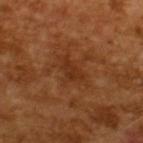Clinical impression:
The lesion was tiled from a total-body skin photograph and was not biopsied.
Acquisition and patient details:
Captured under cross-polarized illumination. A male patient aged 63–67. Measured at roughly 3.5 mm in maximum diameter. A region of skin cropped from a whole-body photographic capture, roughly 15 mm wide. An algorithmic analysis of the crop reported an eccentricity of roughly 0.8 and two-axis asymmetry of about 0.4. The software also gave a border-irregularity rating of about 5/10 and internal color variation of about 1.5 on a 0–10 scale.